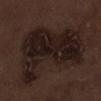Imaged during a routine full-body skin examination; the lesion was not biopsied and no histopathology is available. The patient is a male in their 70s. Automated tile analysis of the lesion measured a lesion color around L≈17 a*≈12 b*≈15 in CIELAB, about 8 CIELAB-L* units darker than the surrounding skin, and a normalized lesion–skin contrast near 11. The software also gave an automated nevus-likeness rating near 0 out of 100 and a detector confidence of about 95 out of 100 that the crop contains a lesion. A lesion tile, about 15 mm wide, cut from a 3D total-body photograph. Captured under white-light illumination. On the right lower leg. About 9.5 mm across.